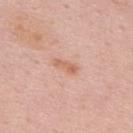Captured during whole-body skin photography for melanoma surveillance; the lesion was not biopsied. The lesion is located on the back. Automated image analysis of the tile measured a lesion area of about 3 mm², an eccentricity of roughly 0.95, and two-axis asymmetry of about 0.35. The software also gave an average lesion color of about L≈63 a*≈24 b*≈31 (CIELAB), about 9 CIELAB-L* units darker than the surrounding skin, and a normalized border contrast of about 6.5. It also reported border irregularity of about 4 on a 0–10 scale and a peripheral color-asymmetry measure near 0. It also reported a lesion-detection confidence of about 100/100. A 15 mm crop from a total-body photograph taken for skin-cancer surveillance. The patient is a male aged approximately 50. Imaged with white-light lighting.